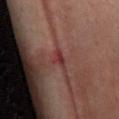Assessment: No biopsy was performed on this lesion — it was imaged during a full skin examination and was not determined to be concerning. Image and clinical context: A 15 mm crop from a total-body photograph taken for skin-cancer surveillance. A female patient, aged around 45. The lesion is located on the chest.